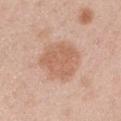{
  "biopsy_status": "not biopsied; imaged during a skin examination",
  "site": "right upper arm",
  "patient": {
    "sex": "male",
    "age_approx": 45
  },
  "lighting": "white-light",
  "image": {
    "source": "total-body photography crop",
    "field_of_view_mm": 15
  }
}